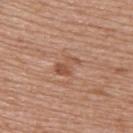image source: 15 mm crop, total-body photography; lighting: white-light; lesion size: ≈3 mm; patient: female, in their 70s; site: the upper back.